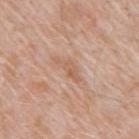Clinical impression:
Captured during whole-body skin photography for melanoma surveillance; the lesion was not biopsied.
Background:
Imaged with white-light lighting. A 15 mm close-up tile from a total-body photography series done for melanoma screening. A male subject aged approximately 50. Located on the upper back.